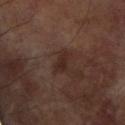{
  "biopsy_status": "not biopsied; imaged during a skin examination",
  "lesion_size": {
    "long_diameter_mm_approx": 3.0
  },
  "site": "left arm",
  "patient": {
    "sex": "female",
    "age_approx": 80
  },
  "image": {
    "source": "total-body photography crop",
    "field_of_view_mm": 15
  }
}